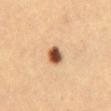Findings:
* workup · imaged on a skin check; not biopsied
* patient · female, aged around 40
* imaging modality · total-body-photography crop, ~15 mm field of view
* location · the front of the torso
* image-analysis metrics · a footprint of about 4 mm² and a symmetry-axis asymmetry near 0.25